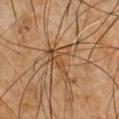{"biopsy_status": "not biopsied; imaged during a skin examination", "lesion_size": {"long_diameter_mm_approx": 5.0}, "image": {"source": "total-body photography crop", "field_of_view_mm": 15}, "site": "chest", "patient": {"sex": "male", "age_approx": 50}, "lighting": "cross-polarized"}